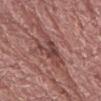workup = catalogued during a skin exam; not biopsied
diameter = about 6 mm
illumination = white-light
patient = male, roughly 70 years of age
location = the right forearm
automated metrics = border irregularity of about 7 on a 0–10 scale, a within-lesion color-variation index near 4/10, and a peripheral color-asymmetry measure near 1; a classifier nevus-likeness of about 0/100
imaging modality = ~15 mm tile from a whole-body skin photo